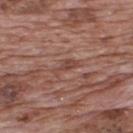Q: Was this lesion biopsied?
A: no biopsy performed (imaged during a skin exam)
Q: Where on the body is the lesion?
A: the upper back
Q: What did automated image analysis measure?
A: an eccentricity of roughly 0.9 and a symmetry-axis asymmetry near 0.45
Q: How was this image acquired?
A: ~15 mm tile from a whole-body skin photo
Q: Illumination type?
A: white-light
Q: Who is the patient?
A: male, about 70 years old